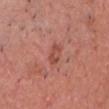Assessment:
Part of a total-body skin-imaging series; this lesion was reviewed on a skin check and was not flagged for biopsy.
Clinical summary:
This image is a 15 mm lesion crop taken from a total-body photograph. The tile uses white-light illumination. Automated image analysis of the tile measured a mean CIELAB color near L≈49 a*≈29 b*≈29 and a normalized border contrast of about 6.5. The software also gave border irregularity of about 6 on a 0–10 scale, a within-lesion color-variation index near 0/10, and a peripheral color-asymmetry measure near 0. The analysis additionally found a classifier nevus-likeness of about 30/100 and a detector confidence of about 100 out of 100 that the crop contains a lesion. About 2.5 mm across. The lesion is on the front of the torso. A male patient, in their 80s.